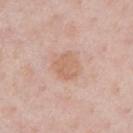The lesion was tiled from a total-body skin photograph and was not biopsied.
About 3 mm across.
A region of skin cropped from a whole-body photographic capture, roughly 15 mm wide.
Imaged with white-light lighting.
From the chest.
A male patient, aged around 60.